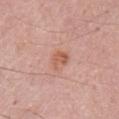Case summary:
- follow-up · no biopsy performed (imaged during a skin exam)
- subject · male, approximately 80 years of age
- image · 15 mm crop, total-body photography
- anatomic site · the front of the torso
- lighting · white-light
- lesion diameter · about 3 mm